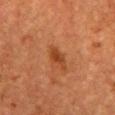– follow-up: imaged on a skin check; not biopsied
– patient: female, approximately 50 years of age
– lesion diameter: about 3 mm
– acquisition: ~15 mm tile from a whole-body skin photo
– image-analysis metrics: a lesion area of about 4 mm², a shape eccentricity near 0.85, and a symmetry-axis asymmetry near 0.3; a classifier nevus-likeness of about 25/100 and a lesion-detection confidence of about 100/100
– illumination: cross-polarized illumination
– body site: the chest